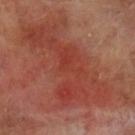The lesion was photographed on a routine skin check and not biopsied; there is no pathology result. On the left lower leg. Automated tile analysis of the lesion measured a nevus-likeness score of about 0/100 and a lesion-detection confidence of about 70/100. This is a cross-polarized tile. A close-up tile cropped from a whole-body skin photograph, about 15 mm across. Approximately 16 mm at its widest. A male subject, in their 70s.